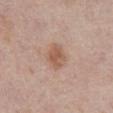Clinical impression:
Captured during whole-body skin photography for melanoma surveillance; the lesion was not biopsied.
Background:
The patient is a male approximately 75 years of age. Automated tile analysis of the lesion measured a classifier nevus-likeness of about 70/100 and a lesion-detection confidence of about 100/100. About 3 mm across. A roughly 15 mm field-of-view crop from a total-body skin photograph. Located on the abdomen. The tile uses white-light illumination.